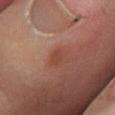biopsy_status: not biopsied; imaged during a skin examination
image:
  source: total-body photography crop
  field_of_view_mm: 15
patient:
  sex: male
  age_approx: 40
site: left lower leg
lighting: cross-polarized
lesion_size:
  long_diameter_mm_approx: 2.0